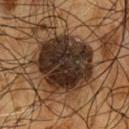follow-up: catalogued during a skin exam; not biopsied | automated metrics: an average lesion color of about L≈27 a*≈14 b*≈22 (CIELAB), about 17 CIELAB-L* units darker than the surrounding skin, and a normalized lesion–skin contrast near 16.5 | lesion size: ~7.5 mm (longest diameter) | acquisition: ~15 mm crop, total-body skin-cancer survey | location: the front of the torso | lighting: cross-polarized illumination | subject: male, roughly 55 years of age.